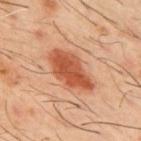Q: Was this lesion biopsied?
A: imaged on a skin check; not biopsied
Q: What is the anatomic site?
A: the upper back
Q: What kind of image is this?
A: total-body-photography crop, ~15 mm field of view
Q: Patient demographics?
A: male, aged around 60